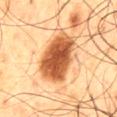<tbp_lesion>
<biopsy_status>not biopsied; imaged during a skin examination</biopsy_status>
<image>
  <source>total-body photography crop</source>
  <field_of_view_mm>15</field_of_view_mm>
</image>
<patient>
  <sex>male</sex>
  <age_approx>60</age_approx>
</patient>
<site>back</site>
<automated_metrics>
  <cielab_L>45</cielab_L>
  <cielab_a>22</cielab_a>
  <cielab_b>34</cielab_b>
  <vs_skin_darker_L>18.0</vs_skin_darker_L>
  <vs_skin_contrast_norm>12.5</vs_skin_contrast_norm>
</automated_metrics>
<lesion_size>
  <long_diameter_mm_approx>8.0</long_diameter_mm_approx>
</lesion_size>
<lighting>cross-polarized</lighting>
</tbp_lesion>